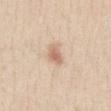Part of a total-body skin-imaging series; this lesion was reviewed on a skin check and was not flagged for biopsy. A region of skin cropped from a whole-body photographic capture, roughly 15 mm wide. This is a white-light tile. The lesion is on the abdomen. A female subject about 40 years old.